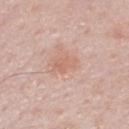<lesion>
  <biopsy_status>not biopsied; imaged during a skin examination</biopsy_status>
  <automated_metrics>
    <cielab_L>63</cielab_L>
    <cielab_a>22</cielab_a>
    <cielab_b>28</cielab_b>
    <vs_skin_darker_L>7.0</vs_skin_darker_L>
    <vs_skin_contrast_norm>5.0</vs_skin_contrast_norm>
    <border_irregularity_0_10>3.0</border_irregularity_0_10>
    <color_variation_0_10>1.5</color_variation_0_10>
    <peripheral_color_asymmetry>0.5</peripheral_color_asymmetry>
    <nevus_likeness_0_100>60</nevus_likeness_0_100>
    <lesion_detection_confidence_0_100>100</lesion_detection_confidence_0_100>
  </automated_metrics>
  <patient>
    <sex>male</sex>
    <age_approx>60</age_approx>
  </patient>
  <lesion_size>
    <long_diameter_mm_approx>3.0</long_diameter_mm_approx>
  </lesion_size>
  <site>chest</site>
  <image>
    <source>total-body photography crop</source>
    <field_of_view_mm>15</field_of_view_mm>
  </image>
</lesion>